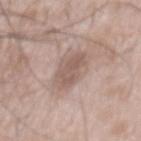This is a white-light tile.
The lesion's longest dimension is about 4.5 mm.
The lesion is on the back.
A male patient aged approximately 50.
A close-up tile cropped from a whole-body skin photograph, about 15 mm across.